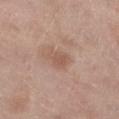Acquisition and patient details:
The subject is a female aged 53–57. Located on the left lower leg. Automated image analysis of the tile measured a border-irregularity rating of about 2.5/10, a within-lesion color-variation index near 2/10, and a peripheral color-asymmetry measure near 0.5. And it measured a classifier nevus-likeness of about 0/100 and a lesion-detection confidence of about 100/100. A lesion tile, about 15 mm wide, cut from a 3D total-body photograph. The tile uses white-light illumination. Approximately 2.5 mm at its widest.